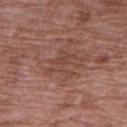| field | value |
|---|---|
| workup | catalogued during a skin exam; not biopsied |
| illumination | white-light illumination |
| patient | female, in their 70s |
| site | the right upper arm |
| image source | ~15 mm crop, total-body skin-cancer survey |
| size | ~4 mm (longest diameter) |